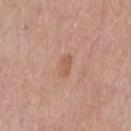follow-up — total-body-photography surveillance lesion; no biopsy
imaging modality — total-body-photography crop, ~15 mm field of view
lighting — white-light illumination
location — the chest
automated lesion analysis — a mean CIELAB color near L≈57 a*≈22 b*≈31 and a normalized lesion–skin contrast near 5.5; border irregularity of about 2 on a 0–10 scale, internal color variation of about 1.5 on a 0–10 scale, and a peripheral color-asymmetry measure near 0.5; lesion-presence confidence of about 100/100
subject — male, in their mid-50s